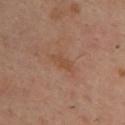{"biopsy_status": "not biopsied; imaged during a skin examination", "site": "upper back", "lighting": "cross-polarized", "image": {"source": "total-body photography crop", "field_of_view_mm": 15}, "patient": {"sex": "male", "age_approx": 40}}